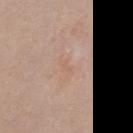– biopsy status — catalogued during a skin exam; not biopsied
– patient — female, aged 53–57
– lesion diameter — about 2 mm
– image source — total-body-photography crop, ~15 mm field of view
– lighting — white-light
– body site — the front of the torso